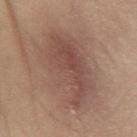Recorded during total-body skin imaging; not selected for excision or biopsy. A female patient, aged 48 to 52. Automated image analysis of the tile measured a border-irregularity index near 3/10, a within-lesion color-variation index near 4.5/10, and radial color variation of about 1.5. It also reported a lesion-detection confidence of about 100/100. Approximately 10 mm at its widest. The lesion is located on the left lower leg. A 15 mm crop from a total-body photograph taken for skin-cancer surveillance. The tile uses cross-polarized illumination.